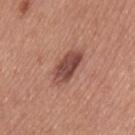location: the left thigh | image: ~15 mm crop, total-body skin-cancer survey | subject: female, roughly 40 years of age | diameter: ~4.5 mm (longest diameter) | automated lesion analysis: a within-lesion color-variation index near 4.5/10.